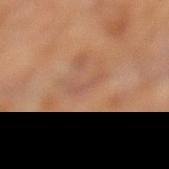Captured during whole-body skin photography for melanoma surveillance; the lesion was not biopsied. The tile uses cross-polarized illumination. The lesion's longest dimension is about 4.5 mm. A female subject, approximately 60 years of age. A close-up tile cropped from a whole-body skin photograph, about 15 mm across. The lesion is located on the left lower leg.